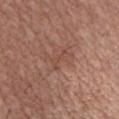notes=catalogued during a skin exam; not biopsied
site=the chest
subject=male, aged 53 to 57
image source=15 mm crop, total-body photography
tile lighting=white-light illumination
image-analysis metrics=an area of roughly 3 mm², a shape eccentricity near 0.9, and a symmetry-axis asymmetry near 0.6; a border-irregularity rating of about 7/10, a color-variation rating of about 0/10, and a peripheral color-asymmetry measure near 0
lesion size=~3 mm (longest diameter)